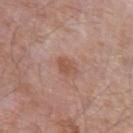notes — imaged on a skin check; not biopsied
patient — male, about 65 years old
anatomic site — the left upper arm
imaging modality — total-body-photography crop, ~15 mm field of view
illumination — white-light